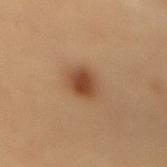The lesion was tiled from a total-body skin photograph and was not biopsied.
Located on the lower back.
Captured under cross-polarized illumination.
The lesion-visualizer software estimated an average lesion color of about L≈37 a*≈18 b*≈28 (CIELAB), roughly 10 lightness units darker than nearby skin, and a normalized border contrast of about 9. It also reported a border-irregularity rating of about 1.5/10 and a peripheral color-asymmetry measure near 1.
A roughly 15 mm field-of-view crop from a total-body skin photograph.
The lesion's longest dimension is about 3.5 mm.
The subject is a male approximately 55 years of age.